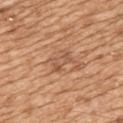A female subject, aged 53–57.
Imaged with white-light lighting.
The lesion is located on the upper back.
A lesion tile, about 15 mm wide, cut from a 3D total-body photograph.